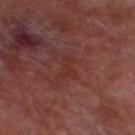{
  "biopsy_status": "not biopsied; imaged during a skin examination",
  "patient": {
    "sex": "male",
    "age_approx": 70
  },
  "lighting": "cross-polarized",
  "image": {
    "source": "total-body photography crop",
    "field_of_view_mm": 15
  },
  "site": "leg"
}